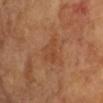Q: Was a biopsy performed?
A: catalogued during a skin exam; not biopsied
Q: Lesion location?
A: the left upper arm
Q: What are the patient's age and sex?
A: female, aged 73–77
Q: How large is the lesion?
A: ≈3 mm
Q: How was this image acquired?
A: 15 mm crop, total-body photography
Q: Automated lesion metrics?
A: a footprint of about 5 mm², an eccentricity of roughly 0.7, and a symmetry-axis asymmetry near 0.5; an automated nevus-likeness rating near 0 out of 100 and lesion-presence confidence of about 100/100